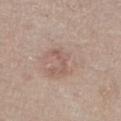– biopsy status: imaged on a skin check; not biopsied
– image source: 15 mm crop, total-body photography
– body site: the right lower leg
– lighting: white-light
– subject: female, approximately 55 years of age